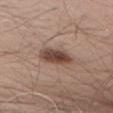• notes: imaged on a skin check; not biopsied
• image: ~15 mm crop, total-body skin-cancer survey
• subject: male, aged around 70
• location: the back
• automated lesion analysis: a footprint of about 8.5 mm², a shape eccentricity near 0.9, and a symmetry-axis asymmetry near 0.2; a mean CIELAB color near L≈45 a*≈17 b*≈25, roughly 14 lightness units darker than nearby skin, and a lesion-to-skin contrast of about 10.5 (normalized; higher = more distinct); a border-irregularity index near 2.5/10, a within-lesion color-variation index near 4.5/10, and peripheral color asymmetry of about 1.5; an automated nevus-likeness rating near 95 out of 100 and a lesion-detection confidence of about 100/100
• tile lighting: white-light